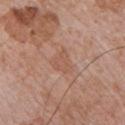Findings:
– biopsy status: no biopsy performed (imaged during a skin exam)
– patient: male, aged 68 to 72
– anatomic site: the front of the torso
– lighting: white-light
– size: ≈3.5 mm
– image source: total-body-photography crop, ~15 mm field of view